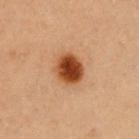notes: imaged on a skin check; not biopsied
illumination: cross-polarized illumination
automated metrics: a lesion area of about 9 mm², an outline eccentricity of about 0.5 (0 = round, 1 = elongated), and two-axis asymmetry of about 0.1; a classifier nevus-likeness of about 100/100 and lesion-presence confidence of about 100/100
lesion diameter: ≈3.5 mm
anatomic site: the chest
acquisition: total-body-photography crop, ~15 mm field of view
patient: female, about 40 years old